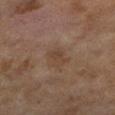Q: Was a biopsy performed?
A: total-body-photography surveillance lesion; no biopsy
Q: What lighting was used for the tile?
A: cross-polarized
Q: Automated lesion metrics?
A: a lesion color around L≈36 a*≈14 b*≈24 in CIELAB and roughly 5 lightness units darker than nearby skin; peripheral color asymmetry of about 0.5; an automated nevus-likeness rating near 0 out of 100 and lesion-presence confidence of about 100/100
Q: What kind of image is this?
A: ~15 mm tile from a whole-body skin photo
Q: Lesion size?
A: ~2.5 mm (longest diameter)
Q: What is the anatomic site?
A: the left lower leg
Q: Patient demographics?
A: female, in their 60s